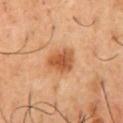Clinical impression: Part of a total-body skin-imaging series; this lesion was reviewed on a skin check and was not flagged for biopsy. Background: From the chest. A 15 mm close-up extracted from a 3D total-body photography capture. The patient is a male roughly 50 years of age.